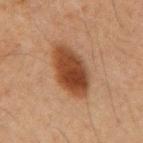{
  "biopsy_status": "not biopsied; imaged during a skin examination"
}